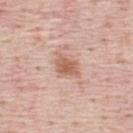<case>
<biopsy_status>not biopsied; imaged during a skin examination</biopsy_status>
<lesion_size>
  <long_diameter_mm_approx>3.0</long_diameter_mm_approx>
</lesion_size>
<site>mid back</site>
<patient>
  <sex>male</sex>
  <age_approx>45</age_approx>
</patient>
<image>
  <source>total-body photography crop</source>
  <field_of_view_mm>15</field_of_view_mm>
</image>
</case>